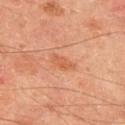body site — the upper back | illumination — cross-polarized illumination | TBP lesion metrics — an average lesion color of about L≈59 a*≈27 b*≈38 (CIELAB) and a lesion-to-skin contrast of about 5.5 (normalized; higher = more distinct); border irregularity of about 3.5 on a 0–10 scale and a peripheral color-asymmetry measure near 1; an automated nevus-likeness rating near 0 out of 100 | subject — male, in their mid-60s | acquisition — ~15 mm tile from a whole-body skin photo | diameter — ~3 mm (longest diameter).